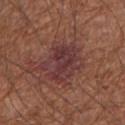workup = no biopsy performed (imaged during a skin exam) | lesion size = about 5.5 mm | imaging modality = total-body-photography crop, ~15 mm field of view | site = the right forearm | illumination = white-light | subject = male, roughly 65 years of age.